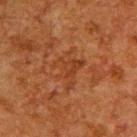<lesion>
  <biopsy_status>not biopsied; imaged during a skin examination</biopsy_status>
</lesion>